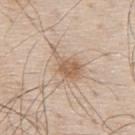Imaged during a routine full-body skin examination; the lesion was not biopsied and no histopathology is available.
Captured under white-light illumination.
The lesion is located on the upper back.
A male subject, roughly 80 years of age.
A roughly 15 mm field-of-view crop from a total-body skin photograph.